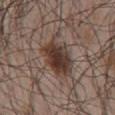Impression:
No biopsy was performed on this lesion — it was imaged during a full skin examination and was not determined to be concerning.
Acquisition and patient details:
The lesion is on the chest. The tile uses white-light illumination. A male subject, roughly 65 years of age. Measured at roughly 5.5 mm in maximum diameter. Cropped from a total-body skin-imaging series; the visible field is about 15 mm.